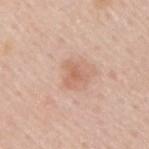Impression: The lesion was photographed on a routine skin check and not biopsied; there is no pathology result. Background: A male patient, aged 58 to 62. The total-body-photography lesion software estimated a footprint of about 4.5 mm², a shape eccentricity near 0.5, and a symmetry-axis asymmetry near 0.4. The software also gave a lesion–skin lightness drop of about 8 and a normalized lesion–skin contrast near 5.5. And it measured border irregularity of about 4 on a 0–10 scale and a peripheral color-asymmetry measure near 0.5. It also reported a nevus-likeness score of about 5/100 and a lesion-detection confidence of about 100/100. This image is a 15 mm lesion crop taken from a total-body photograph. The lesion is located on the arm.